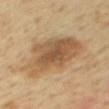Q: Was a biopsy performed?
A: total-body-photography surveillance lesion; no biopsy
Q: Lesion size?
A: ~8 mm (longest diameter)
Q: Who is the patient?
A: female, in their mid-50s
Q: Automated lesion metrics?
A: a lesion area of about 26 mm² and a shape-asymmetry score of about 0.3 (0 = symmetric); a classifier nevus-likeness of about 70/100
Q: Where on the body is the lesion?
A: the back
Q: Illumination type?
A: cross-polarized illumination
Q: What is the imaging modality?
A: ~15 mm tile from a whole-body skin photo